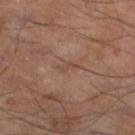Clinical impression: Recorded during total-body skin imaging; not selected for excision or biopsy. Background: Located on the left lower leg. An algorithmic analysis of the crop reported a lesion area of about 2.5 mm². The software also gave roughly 5 lightness units darker than nearby skin and a normalized lesion–skin contrast near 4.5. It also reported a border-irregularity index near 5.5/10 and radial color variation of about 0. A male subject, aged 53–57. A 15 mm close-up tile from a total-body photography series done for melanoma screening. The lesion's longest dimension is about 2.5 mm.